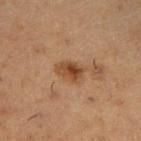image:
  source: total-body photography crop
  field_of_view_mm: 15
site: right upper arm
patient:
  sex: male
  age_approx: 55